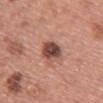{
  "biopsy_status": "not biopsied; imaged during a skin examination",
  "image": {
    "source": "total-body photography crop",
    "field_of_view_mm": 15
  },
  "patient": {
    "sex": "female",
    "age_approx": 60
  },
  "site": "chest"
}